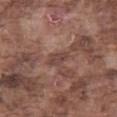The lesion was photographed on a routine skin check and not biopsied; there is no pathology result.
This is a white-light tile.
A 15 mm close-up tile from a total-body photography series done for melanoma screening.
A male subject, aged around 75.
An algorithmic analysis of the crop reported an outline eccentricity of about 0.85 (0 = round, 1 = elongated) and a shape-asymmetry score of about 0.25 (0 = symmetric). And it measured a mean CIELAB color near L≈42 a*≈19 b*≈23 and roughly 8 lightness units darker than nearby skin. The analysis additionally found a color-variation rating of about 1.5/10 and a peripheral color-asymmetry measure near 0.5.
The lesion is located on the left thigh.